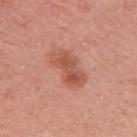Recorded during total-body skin imaging; not selected for excision or biopsy. From the right upper arm. The recorded lesion diameter is about 5 mm. A 15 mm close-up tile from a total-body photography series done for melanoma screening. The tile uses white-light illumination. A female subject aged 48 to 52. An algorithmic analysis of the crop reported a border-irregularity index near 3/10 and peripheral color asymmetry of about 1.5.